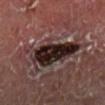Impression: This lesion was catalogued during total-body skin photography and was not selected for biopsy. Image and clinical context: Cropped from a total-body skin-imaging series; the visible field is about 15 mm. The patient is a male aged 68 to 72. Imaged with cross-polarized lighting. The total-body-photography lesion software estimated a footprint of about 21 mm², an outline eccentricity of about 0.85 (0 = round, 1 = elongated), and a symmetry-axis asymmetry near 0.35. The software also gave border irregularity of about 5 on a 0–10 scale and a peripheral color-asymmetry measure near 2.5. The software also gave an automated nevus-likeness rating near 0 out of 100 and lesion-presence confidence of about 100/100. The lesion is on the right lower leg.